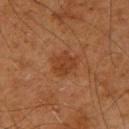Impression: No biopsy was performed on this lesion — it was imaged during a full skin examination and was not determined to be concerning. Acquisition and patient details: A close-up tile cropped from a whole-body skin photograph, about 15 mm across. Imaged with cross-polarized lighting. The lesion is on the left upper arm. A male subject aged approximately 60. Measured at roughly 3 mm in maximum diameter.